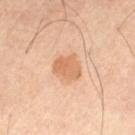biopsy status: total-body-photography surveillance lesion; no biopsy | location: the left thigh | image: ~15 mm tile from a whole-body skin photo | illumination: cross-polarized | patient: male, aged 63 to 67 | size: about 3 mm.